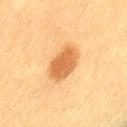{"biopsy_status": "not biopsied; imaged during a skin examination", "patient": {"sex": "female", "age_approx": 55}, "image": {"source": "total-body photography crop", "field_of_view_mm": 15}, "automated_metrics": {"area_mm2_approx": 11.0, "eccentricity": 0.8, "nevus_likeness_0_100": 100, "lesion_detection_confidence_0_100": 100}, "lesion_size": {"long_diameter_mm_approx": 4.5}, "site": "back"}